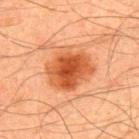{"biopsy_status": "not biopsied; imaged during a skin examination", "patient": {"sex": "male", "age_approx": 45}, "lighting": "cross-polarized", "image": {"source": "total-body photography crop", "field_of_view_mm": 15}, "lesion_size": {"long_diameter_mm_approx": 5.5}, "site": "upper back"}